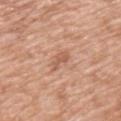biopsy status=imaged on a skin check; not biopsied | image source=15 mm crop, total-body photography | subject=male, aged approximately 50 | automated lesion analysis=a footprint of about 3 mm² and two-axis asymmetry of about 0.45 | location=the upper back | lighting=white-light illumination.